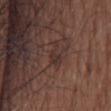– workup · total-body-photography surveillance lesion; no biopsy
– anatomic site · the head or neck
– size · ≈3.5 mm
– image · ~15 mm tile from a whole-body skin photo
– tile lighting · white-light
– subject · female, in their mid- to late 70s